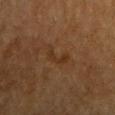<case>
<biopsy_status>not biopsied; imaged during a skin examination</biopsy_status>
<site>right upper arm</site>
<patient>
  <sex>female</sex>
  <age_approx>55</age_approx>
</patient>
<image>
  <source>total-body photography crop</source>
  <field_of_view_mm>15</field_of_view_mm>
</image>
</case>